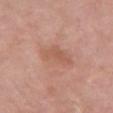workup: total-body-photography surveillance lesion; no biopsy | lesion diameter: ~5 mm (longest diameter) | automated lesion analysis: a footprint of about 11 mm², an outline eccentricity of about 0.85 (0 = round, 1 = elongated), and a shape-asymmetry score of about 0.2 (0 = symmetric); a border-irregularity rating of about 2.5/10, a within-lesion color-variation index near 2.5/10, and radial color variation of about 1; an automated nevus-likeness rating near 0 out of 100 and a lesion-detection confidence of about 100/100 | site: the upper back | patient: female, approximately 65 years of age | image: ~15 mm tile from a whole-body skin photo.